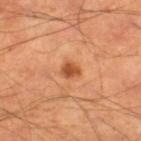subject = male, in their mid-50s; lesion size = ≈2.5 mm; image source = 15 mm crop, total-body photography; illumination = cross-polarized; location = the right lower leg.